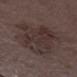Clinical impression: Captured during whole-body skin photography for melanoma surveillance; the lesion was not biopsied. Background: The lesion is located on the left thigh. Approximately 8.5 mm at its widest. The total-body-photography lesion software estimated a border-irregularity rating of about 4.5/10, a within-lesion color-variation index near 4.5/10, and a peripheral color-asymmetry measure near 1.5. And it measured lesion-presence confidence of about 100/100. A roughly 15 mm field-of-view crop from a total-body skin photograph. The tile uses white-light illumination. A male patient, aged approximately 70.